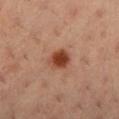biopsy_status: not biopsied; imaged during a skin examination
site: left lower leg
lighting: cross-polarized
automated_metrics:
  area_mm2_approx: 5.5
  eccentricity: 0.6
  shape_asymmetry: 0.2
  cielab_L: 44
  cielab_a: 26
  cielab_b: 34
  vs_skin_darker_L: 15.0
  vs_skin_contrast_norm: 11.5
  border_irregularity_0_10: 1.5
  color_variation_0_10: 3.0
  nevus_likeness_0_100: 100
  lesion_detection_confidence_0_100: 100
image:
  source: total-body photography crop
  field_of_view_mm: 15
patient:
  sex: female
  age_approx: 30
lesion_size:
  long_diameter_mm_approx: 3.0